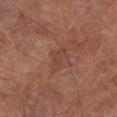follow-up = imaged on a skin check; not biopsied
location = the right lower leg
size = ≈3 mm
patient = male, aged approximately 75
illumination = cross-polarized illumination
image source = total-body-photography crop, ~15 mm field of view